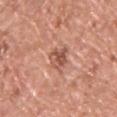<record>
<biopsy_status>not biopsied; imaged during a skin examination</biopsy_status>
<site>chest</site>
<patient>
  <sex>male</sex>
  <age_approx>55</age_approx>
</patient>
<automated_metrics>
  <area_mm2_approx>6.0</area_mm2_approx>
  <eccentricity>0.6</eccentricity>
</automated_metrics>
<image>
  <source>total-body photography crop</source>
  <field_of_view_mm>15</field_of_view_mm>
</image>
<lighting>white-light</lighting>
</record>